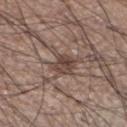notes — imaged on a skin check; not biopsied | lighting — white-light illumination | patient — male, in their mid- to late 30s | size — ~4 mm (longest diameter) | location — the leg | acquisition — ~15 mm tile from a whole-body skin photo.